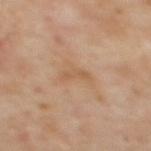The tile uses cross-polarized illumination.
An algorithmic analysis of the crop reported an average lesion color of about L≈56 a*≈19 b*≈34 (CIELAB) and a normalized border contrast of about 5. The software also gave a border-irregularity index near 4.5/10, a within-lesion color-variation index near 0/10, and peripheral color asymmetry of about 0. It also reported an automated nevus-likeness rating near 0 out of 100 and lesion-presence confidence of about 100/100.
On the back.
Cropped from a whole-body photographic skin survey; the tile spans about 15 mm.
A female subject, aged around 60.